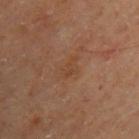notes: total-body-photography surveillance lesion; no biopsy
diameter: about 2.5 mm
subject: male, in their mid-60s
automated lesion analysis: a footprint of about 3.5 mm², an outline eccentricity of about 0.8 (0 = round, 1 = elongated), and a symmetry-axis asymmetry near 0.45; a mean CIELAB color near L≈42 a*≈20 b*≈31 and a normalized lesion–skin contrast near 4.5; a classifier nevus-likeness of about 0/100 and a lesion-detection confidence of about 100/100
illumination: cross-polarized illumination
acquisition: ~15 mm crop, total-body skin-cancer survey
anatomic site: the upper back